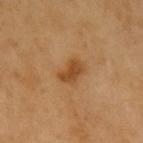Longest diameter approximately 3 mm.
The tile uses cross-polarized illumination.
The patient is a male aged approximately 60.
The total-body-photography lesion software estimated an area of roughly 5.5 mm², a shape eccentricity near 0.8, and two-axis asymmetry of about 0.25. And it measured a mean CIELAB color near L≈46 a*≈22 b*≈39, a lesion–skin lightness drop of about 10, and a normalized border contrast of about 8. The software also gave a border-irregularity rating of about 2.5/10, internal color variation of about 2 on a 0–10 scale, and radial color variation of about 0.5. The analysis additionally found lesion-presence confidence of about 100/100.
A 15 mm close-up tile from a total-body photography series done for melanoma screening.
The lesion is located on the left upper arm.